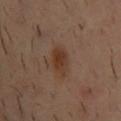{"biopsy_status": "not biopsied; imaged during a skin examination", "lighting": "cross-polarized", "image": {"source": "total-body photography crop", "field_of_view_mm": 15}, "patient": {"sex": "male", "age_approx": 40}, "site": "upper back", "lesion_size": {"long_diameter_mm_approx": 3.5}}